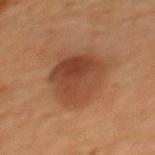Automated image analysis of the tile measured an area of roughly 21 mm², an outline eccentricity of about 0.45 (0 = round, 1 = elongated), and a symmetry-axis asymmetry near 0.2. And it measured a border-irregularity index near 2/10, a within-lesion color-variation index near 6.5/10, and a peripheral color-asymmetry measure near 2.5. And it measured an automated nevus-likeness rating near 65 out of 100.
Cropped from a whole-body photographic skin survey; the tile spans about 15 mm.
On the upper back.
The recorded lesion diameter is about 5.5 mm.
A female patient aged 43 to 47.
Imaged with cross-polarized lighting.